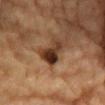follow-up: imaged on a skin check; not biopsied | image source: 15 mm crop, total-body photography | patient: male, in their mid- to late 80s or older | body site: the chest.